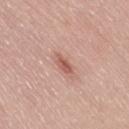Imaged during a routine full-body skin examination; the lesion was not biopsied and no histopathology is available.
Located on the right thigh.
A female patient about 65 years old.
A lesion tile, about 15 mm wide, cut from a 3D total-body photograph.